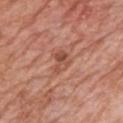Recorded during total-body skin imaging; not selected for excision or biopsy.